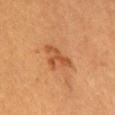  biopsy_status: not biopsied; imaged during a skin examination
  automated_metrics:
    area_mm2_approx: 6.0
    eccentricity: 0.8
    shape_asymmetry: 0.55
    cielab_L: 44
    cielab_a: 23
    cielab_b: 36
    vs_skin_contrast_norm: 6.5
    border_irregularity_0_10: 7.0
    peripheral_color_asymmetry: 0.0
    nevus_likeness_0_100: 15
  site: mid back
  lesion_size:
    long_diameter_mm_approx: 4.0
  lighting: cross-polarized
  patient:
    sex: female
    age_approx: 55
  image:
    source: total-body photography crop
    field_of_view_mm: 15